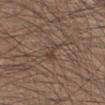The lesion is on the right lower leg. Automated tile analysis of the lesion measured a border-irregularity index near 5/10 and a peripheral color-asymmetry measure near 0. The software also gave an automated nevus-likeness rating near 0 out of 100. About 3 mm across. The tile uses white-light illumination. A 15 mm close-up extracted from a 3D total-body photography capture. A male patient, aged 43–47.